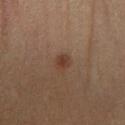Findings:
- biopsy status · imaged on a skin check; not biopsied
- acquisition · ~15 mm crop, total-body skin-cancer survey
- tile lighting · cross-polarized
- patient · male, aged around 60
- lesion size · ~2 mm (longest diameter)
- anatomic site · the leg